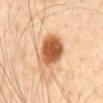This lesion was catalogued during total-body skin photography and was not selected for biopsy. On the abdomen. A male subject, aged 43–47. This is a cross-polarized tile. A close-up tile cropped from a whole-body skin photograph, about 15 mm across.